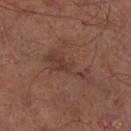Notes:
– notes — catalogued during a skin exam; not biopsied
– imaging modality — ~15 mm tile from a whole-body skin photo
– lesion diameter — ~6.5 mm (longest diameter)
– body site — the left lower leg
– TBP lesion metrics — a lesion color around L≈37 a*≈19 b*≈23 in CIELAB, roughly 6 lightness units darker than nearby skin, and a normalized lesion–skin contrast near 5.5
– patient — male, roughly 65 years of age